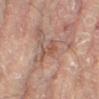Case summary:
- notes: imaged on a skin check; not biopsied
- anatomic site: the left leg
- image-analysis metrics: a border-irregularity index near 3.5/10; a classifier nevus-likeness of about 0/100 and a lesion-detection confidence of about 95/100
- diameter: about 2.5 mm
- acquisition: 15 mm crop, total-body photography
- patient: female, roughly 80 years of age